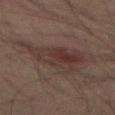Captured during whole-body skin photography for melanoma surveillance; the lesion was not biopsied.
The tile uses cross-polarized illumination.
Automated tile analysis of the lesion measured an outline eccentricity of about 0.8 (0 = round, 1 = elongated). It also reported a mean CIELAB color near L≈27 a*≈13 b*≈17, a lesion–skin lightness drop of about 6, and a normalized lesion–skin contrast near 6.5. It also reported a border-irregularity index near 5.5/10, a color-variation rating of about 5/10, and a peripheral color-asymmetry measure near 2. And it measured an automated nevus-likeness rating near 15 out of 100 and a lesion-detection confidence of about 100/100.
The recorded lesion diameter is about 7.5 mm.
A close-up tile cropped from a whole-body skin photograph, about 15 mm across.
Located on the front of the torso.
The subject is a male aged approximately 45.